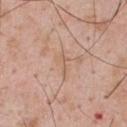Notes:
* notes — catalogued during a skin exam; not biopsied
* image — ~15 mm crop, total-body skin-cancer survey
* site — the upper back
* illumination — white-light
* lesion diameter — about 3 mm
* subject — male, aged 53 to 57